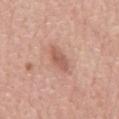Impression:
This lesion was catalogued during total-body skin photography and was not selected for biopsy.
Context:
A male patient, aged 73 to 77. A lesion tile, about 15 mm wide, cut from a 3D total-body photograph. Captured under white-light illumination. The lesion-visualizer software estimated a footprint of about 5 mm², a shape eccentricity near 0.85, and two-axis asymmetry of about 0.25. It also reported a mean CIELAB color near L≈57 a*≈24 b*≈28, a lesion–skin lightness drop of about 10, and a lesion-to-skin contrast of about 7 (normalized; higher = more distinct). The software also gave a border-irregularity index near 2.5/10. The lesion is located on the back.